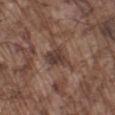Captured during whole-body skin photography for melanoma surveillance; the lesion was not biopsied. A male subject aged 73 to 77. The lesion is on the left lower leg. A 15 mm close-up tile from a total-body photography series done for melanoma screening.